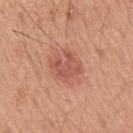Imaged during a routine full-body skin examination; the lesion was not biopsied and no histopathology is available.
On the right upper arm.
Cropped from a total-body skin-imaging series; the visible field is about 15 mm.
The patient is a male aged 18 to 22.
The tile uses white-light illumination.